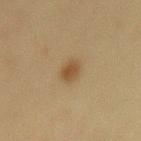{"patient": {"sex": "female", "age_approx": 50}, "image": {"source": "total-body photography crop", "field_of_view_mm": 15}, "site": "mid back", "lighting": "cross-polarized"}